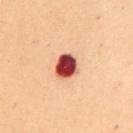Clinical impression:
Part of a total-body skin-imaging series; this lesion was reviewed on a skin check and was not flagged for biopsy.